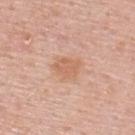{"biopsy_status": "not biopsied; imaged during a skin examination", "automated_metrics": {"nevus_likeness_0_100": 15, "lesion_detection_confidence_0_100": 100}, "image": {"source": "total-body photography crop", "field_of_view_mm": 15}, "patient": {"sex": "male", "age_approx": 55}, "site": "upper back"}